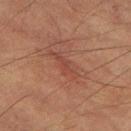Q: Was this lesion biopsied?
A: total-body-photography surveillance lesion; no biopsy
Q: Lesion location?
A: the left thigh
Q: How was this image acquired?
A: ~15 mm tile from a whole-body skin photo
Q: What are the patient's age and sex?
A: male, approximately 85 years of age or older